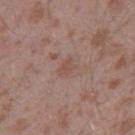Clinical impression:
No biopsy was performed on this lesion — it was imaged during a full skin examination and was not determined to be concerning.
Context:
A male patient, aged around 45. About 2.5 mm across. A region of skin cropped from a whole-body photographic capture, roughly 15 mm wide. Imaged with white-light lighting. The lesion is on the right thigh.